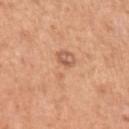Q: Is there a histopathology result?
A: no biopsy performed (imaged during a skin exam)
Q: What is the imaging modality?
A: ~15 mm tile from a whole-body skin photo
Q: Who is the patient?
A: male, roughly 55 years of age
Q: Automated lesion metrics?
A: a footprint of about 9 mm², an eccentricity of roughly 0.9, and two-axis asymmetry of about 0.5; a lesion–skin lightness drop of about 7 and a normalized border contrast of about 4.5; a border-irregularity rating of about 6/10, a within-lesion color-variation index near 5/10, and peripheral color asymmetry of about 2; a classifier nevus-likeness of about 0/100 and lesion-presence confidence of about 100/100
Q: Lesion location?
A: the left upper arm